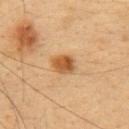patient — female, roughly 30 years of age; body site — the upper back; tile lighting — cross-polarized illumination; acquisition — ~15 mm crop, total-body skin-cancer survey.